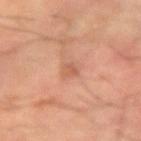Recorded during total-body skin imaging; not selected for excision or biopsy. On the right forearm. About 2.5 mm across. This image is a 15 mm lesion crop taken from a total-body photograph. A male patient approximately 60 years of age.